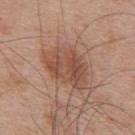Part of a total-body skin-imaging series; this lesion was reviewed on a skin check and was not flagged for biopsy.
From the upper back.
About 6 mm across.
A roughly 15 mm field-of-view crop from a total-body skin photograph.
This is a white-light tile.
The total-body-photography lesion software estimated a lesion area of about 17 mm², an outline eccentricity of about 0.75 (0 = round, 1 = elongated), and a symmetry-axis asymmetry near 0.25. And it measured about 9 CIELAB-L* units darker than the surrounding skin and a normalized border contrast of about 7. The analysis additionally found a border-irregularity rating of about 3/10, a color-variation rating of about 4.5/10, and peripheral color asymmetry of about 1.5. And it measured a nevus-likeness score of about 50/100 and lesion-presence confidence of about 100/100.
A male subject, aged 53 to 57.